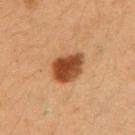Imaged during a routine full-body skin examination; the lesion was not biopsied and no histopathology is available. About 4.5 mm across. Imaged with cross-polarized lighting. A female patient aged around 40. A 15 mm crop from a total-body photograph taken for skin-cancer surveillance. The lesion is on the arm.